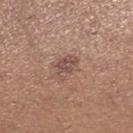Part of a total-body skin-imaging series; this lesion was reviewed on a skin check and was not flagged for biopsy.
A female patient, aged 28 to 32.
The lesion is on the right lower leg.
Cropped from a whole-body photographic skin survey; the tile spans about 15 mm.